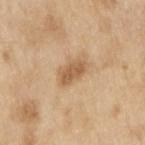This lesion was catalogued during total-body skin photography and was not selected for biopsy.